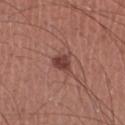{"biopsy_status": "not biopsied; imaged during a skin examination", "patient": {"sex": "male", "age_approx": 45}, "image": {"source": "total-body photography crop", "field_of_view_mm": 15}, "site": "left lower leg"}